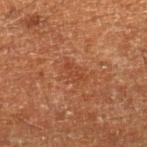Case summary:
- follow-up: imaged on a skin check; not biopsied
- TBP lesion metrics: an area of roughly 5 mm², an outline eccentricity of about 0.75 (0 = round, 1 = elongated), and a symmetry-axis asymmetry near 0.25; a within-lesion color-variation index near 1.5/10 and a peripheral color-asymmetry measure near 0.5; an automated nevus-likeness rating near 0 out of 100 and lesion-presence confidence of about 100/100
- site: the right lower leg
- subject: male, in their 60s
- imaging modality: 15 mm crop, total-body photography